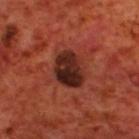| field | value |
|---|---|
| follow-up | catalogued during a skin exam; not biopsied |
| site | the back |
| patient | male, aged 68–72 |
| diameter | about 4.5 mm |
| image source | total-body-photography crop, ~15 mm field of view |